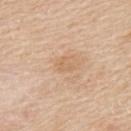Imaged during a routine full-body skin examination; the lesion was not biopsied and no histopathology is available. The lesion is on the upper back. A 15 mm close-up tile from a total-body photography series done for melanoma screening. A male patient, about 60 years old. The total-body-photography lesion software estimated an average lesion color of about L≈64 a*≈18 b*≈35 (CIELAB), a lesion–skin lightness drop of about 6, and a normalized lesion–skin contrast near 4. Longest diameter approximately 2 mm. Captured under white-light illumination.